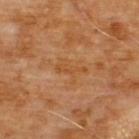Notes:
* notes · no biopsy performed (imaged during a skin exam)
* lighting · cross-polarized illumination
* site · the chest
* image · ~15 mm crop, total-body skin-cancer survey
* size · ≈3.5 mm
* patient · male, aged approximately 60
* image-analysis metrics · a lesion-detection confidence of about 100/100